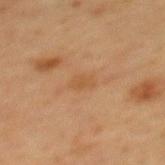<record>
<biopsy_status>not biopsied; imaged during a skin examination</biopsy_status>
<image>
  <source>total-body photography crop</source>
  <field_of_view_mm>15</field_of_view_mm>
</image>
<site>mid back</site>
<patient>
  <sex>female</sex>
  <age_approx>50</age_approx>
</patient>
</record>